The recorded lesion diameter is about 13.5 mm. Cropped from a total-body skin-imaging series; the visible field is about 15 mm. A male patient aged 58 to 62. On the upper back. Imaged with cross-polarized lighting.
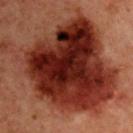Histopathological examination showed a melanoma in situ — a malignancy.